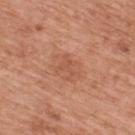– follow-up · catalogued during a skin exam; not biopsied
– patient · male, aged around 70
– size · ~2.5 mm (longest diameter)
– image source · total-body-photography crop, ~15 mm field of view
– illumination · white-light
– body site · the upper back
– TBP lesion metrics · a footprint of about 4 mm², an eccentricity of roughly 0.65, and two-axis asymmetry of about 0.5; a mean CIELAB color near L≈54 a*≈25 b*≈33, a lesion–skin lightness drop of about 6, and a normalized border contrast of about 4.5; a border-irregularity rating of about 6/10, a within-lesion color-variation index near 1/10, and radial color variation of about 0.5